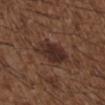This lesion was catalogued during total-body skin photography and was not selected for biopsy.
A male subject, aged 48–52.
On the back.
An algorithmic analysis of the crop reported an area of roughly 12 mm² and a shape-asymmetry score of about 0.25 (0 = symmetric). It also reported about 9 CIELAB-L* units darker than the surrounding skin. The software also gave a border-irregularity index near 3/10, a color-variation rating of about 4/10, and peripheral color asymmetry of about 1. And it measured a classifier nevus-likeness of about 70/100.
This is a white-light tile.
A 15 mm close-up extracted from a 3D total-body photography capture.
Measured at roughly 4.5 mm in maximum diameter.